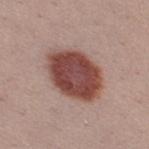Image and clinical context: This is a white-light tile. A female patient about 25 years old. The lesion is on the right thigh. A region of skin cropped from a whole-body photographic capture, roughly 15 mm wide.